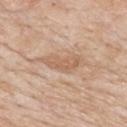No biopsy was performed on this lesion — it was imaged during a full skin examination and was not determined to be concerning. A male subject in their mid-80s or older. Imaged with white-light lighting. A close-up tile cropped from a whole-body skin photograph, about 15 mm across. The lesion is on the upper back. The recorded lesion diameter is about 4.5 mm.